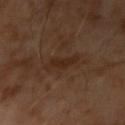No biopsy was performed on this lesion — it was imaged during a full skin examination and was not determined to be concerning. About 3.5 mm across. The subject is a male approximately 65 years of age. Automated image analysis of the tile measured a shape eccentricity near 0.8 and a symmetry-axis asymmetry near 0.35. The software also gave a lesion–skin lightness drop of about 5 and a lesion-to-skin contrast of about 6.5 (normalized; higher = more distinct). The analysis additionally found a classifier nevus-likeness of about 0/100 and a lesion-detection confidence of about 100/100. A 15 mm close-up extracted from a 3D total-body photography capture.